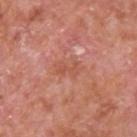Q: Is there a histopathology result?
A: catalogued during a skin exam; not biopsied
Q: Lesion size?
A: ≈3.5 mm
Q: What kind of image is this?
A: 15 mm crop, total-body photography
Q: Who is the patient?
A: male, aged approximately 65
Q: Where on the body is the lesion?
A: the back